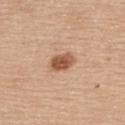biopsy status: no biopsy performed (imaged during a skin exam) | image source: 15 mm crop, total-body photography | lighting: white-light illumination | patient: female, aged 63–67 | body site: the upper back.